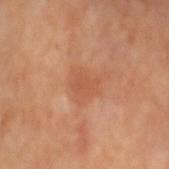Captured during whole-body skin photography for melanoma surveillance; the lesion was not biopsied. The lesion's longest dimension is about 2.5 mm. A female patient, aged 68–72. On the arm. Captured under cross-polarized illumination. A 15 mm close-up extracted from a 3D total-body photography capture.